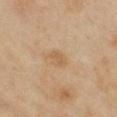workup: imaged on a skin check; not biopsied
patient: female, about 40 years old
automated metrics: an average lesion color of about L≈60 a*≈17 b*≈37 (CIELAB) and a normalized lesion–skin contrast near 5.5; lesion-presence confidence of about 100/100
body site: the chest
imaging modality: ~15 mm crop, total-body skin-cancer survey
tile lighting: cross-polarized illumination